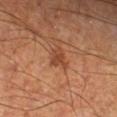{"biopsy_status": "not biopsied; imaged during a skin examination", "automated_metrics": {"vs_skin_contrast_norm": 6.5}, "lighting": "cross-polarized", "patient": {"sex": "male", "age_approx": 65}, "lesion_size": {"long_diameter_mm_approx": 3.0}, "image": {"source": "total-body photography crop", "field_of_view_mm": 15}, "site": "right lower leg"}